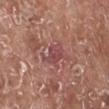Q: Was this lesion biopsied?
A: total-body-photography surveillance lesion; no biopsy
Q: What did automated image analysis measure?
A: a lesion area of about 5.5 mm² and an outline eccentricity of about 0.75 (0 = round, 1 = elongated); a mean CIELAB color near L≈47 a*≈26 b*≈21 and a lesion–skin lightness drop of about 8
Q: What are the patient's age and sex?
A: male, approximately 75 years of age
Q: What lighting was used for the tile?
A: white-light illumination
Q: What kind of image is this?
A: ~15 mm crop, total-body skin-cancer survey
Q: What is the anatomic site?
A: the right lower leg